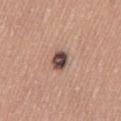Q: Is there a histopathology result?
A: total-body-photography surveillance lesion; no biopsy
Q: How large is the lesion?
A: ~3 mm (longest diameter)
Q: What kind of image is this?
A: ~15 mm tile from a whole-body skin photo
Q: Illumination type?
A: white-light illumination
Q: Where on the body is the lesion?
A: the left thigh
Q: Patient demographics?
A: female, aged 63 to 67